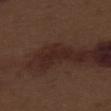Q: Was a biopsy performed?
A: catalogued during a skin exam; not biopsied
Q: What is the anatomic site?
A: the right thigh
Q: What lighting was used for the tile?
A: white-light
Q: Lesion size?
A: about 4 mm
Q: What is the imaging modality?
A: ~15 mm crop, total-body skin-cancer survey
Q: Patient demographics?
A: male, aged approximately 70